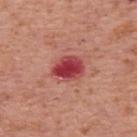* patient · male, aged around 70
* body site · the upper back
* image source · ~15 mm tile from a whole-body skin photo